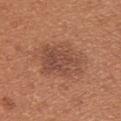biopsy status = no biopsy performed (imaged during a skin exam) | body site = the back | patient = female, aged around 30 | tile lighting = white-light | size = about 6.5 mm | image source = ~15 mm tile from a whole-body skin photo.